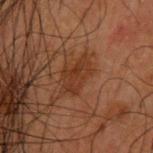Recorded during total-body skin imaging; not selected for excision or biopsy. An algorithmic analysis of the crop reported a shape eccentricity near 0.85 and two-axis asymmetry of about 0.3. It also reported a border-irregularity rating of about 4/10, internal color variation of about 2.5 on a 0–10 scale, and a peripheral color-asymmetry measure near 1. And it measured a classifier nevus-likeness of about 0/100 and lesion-presence confidence of about 100/100. Located on the upper back. The tile uses cross-polarized illumination. A 15 mm close-up tile from a total-body photography series done for melanoma screening. A male patient aged 48 to 52.